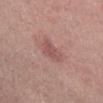Imaged during a routine full-body skin examination; the lesion was not biopsied and no histopathology is available.
The total-body-photography lesion software estimated a mean CIELAB color near L≈52 a*≈23 b*≈22, a lesion–skin lightness drop of about 8, and a normalized lesion–skin contrast near 5.5. It also reported internal color variation of about 2 on a 0–10 scale and a peripheral color-asymmetry measure near 0.5. The analysis additionally found lesion-presence confidence of about 100/100.
A female subject, aged around 40.
Located on the abdomen.
A 15 mm close-up extracted from a 3D total-body photography capture.
Longest diameter approximately 3 mm.